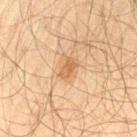Clinical impression: No biopsy was performed on this lesion — it was imaged during a full skin examination and was not determined to be concerning. Background: A male subject aged 58–62. The lesion is located on the left thigh. The tile uses cross-polarized illumination. A roughly 15 mm field-of-view crop from a total-body skin photograph. The lesion-visualizer software estimated a lesion area of about 3.5 mm², an outline eccentricity of about 0.8 (0 = round, 1 = elongated), and a symmetry-axis asymmetry near 0.25. It also reported a lesion–skin lightness drop of about 8 and a normalized lesion–skin contrast near 6.5. The software also gave border irregularity of about 2.5 on a 0–10 scale and internal color variation of about 2 on a 0–10 scale. The analysis additionally found a classifier nevus-likeness of about 45/100 and a detector confidence of about 100 out of 100 that the crop contains a lesion. Measured at roughly 2.5 mm in maximum diameter.